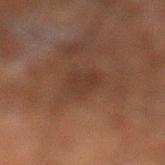illumination = cross-polarized | site = the left lower leg | image-analysis metrics = a lesion area of about 7.5 mm² and a symmetry-axis asymmetry near 0.25; a border-irregularity rating of about 2.5/10; an automated nevus-likeness rating near 15 out of 100 and a lesion-detection confidence of about 100/100 | subject = male, aged around 50 | lesion diameter = ≈3.5 mm | imaging modality = total-body-photography crop, ~15 mm field of view.